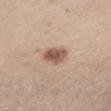follow-up — total-body-photography surveillance lesion; no biopsy
acquisition — ~15 mm tile from a whole-body skin photo
diameter — about 3 mm
site — the chest
automated metrics — an average lesion color of about L≈55 a*≈18 b*≈28 (CIELAB) and about 14 CIELAB-L* units darker than the surrounding skin; a nevus-likeness score of about 90/100 and lesion-presence confidence of about 100/100
patient — female, aged approximately 65